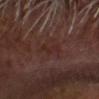follow-up: total-body-photography surveillance lesion; no biopsy
automated metrics: a shape eccentricity near 0.9 and two-axis asymmetry of about 0.4; a mean CIELAB color near L≈25 a*≈19 b*≈20 and a lesion-to-skin contrast of about 5.5 (normalized; higher = more distinct)
lighting: cross-polarized
subject: male, in their mid- to late 60s
image: 15 mm crop, total-body photography